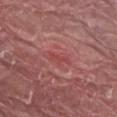Clinical impression:
The lesion was photographed on a routine skin check and not biopsied; there is no pathology result.
Image and clinical context:
Located on the abdomen. The tile uses white-light illumination. Automated image analysis of the tile measured lesion-presence confidence of about 85/100. The subject is a male aged 38–42. The lesion's longest dimension is about 3 mm. A 15 mm close-up tile from a total-body photography series done for melanoma screening.